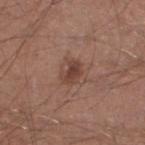Recorded during total-body skin imaging; not selected for excision or biopsy. Cropped from a total-body skin-imaging series; the visible field is about 15 mm. The patient is a male in their 30s. From the left lower leg.